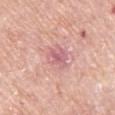– biopsy status: no biopsy performed (imaged during a skin exam)
– diameter: about 3.5 mm
– location: the left upper arm
– acquisition: ~15 mm crop, total-body skin-cancer survey
– illumination: white-light
– subject: female, aged 63 to 67